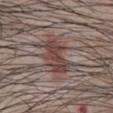Assessment: The lesion was photographed on a routine skin check and not biopsied; there is no pathology result. Clinical summary: From the abdomen. A 15 mm close-up tile from a total-body photography series done for melanoma screening. A male subject, roughly 40 years of age. The tile uses white-light illumination.